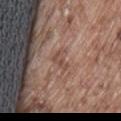notes: imaged on a skin check; not biopsied | patient: male, aged around 75 | image source: 15 mm crop, total-body photography | TBP lesion metrics: a lesion area of about 3.5 mm² and a shape eccentricity near 0.8; an average lesion color of about L≈49 a*≈18 b*≈25 (CIELAB) and about 7 CIELAB-L* units darker than the surrounding skin; border irregularity of about 5.5 on a 0–10 scale and internal color variation of about 2 on a 0–10 scale | tile lighting: white-light | location: the lower back.